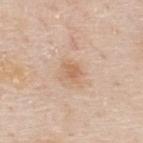Q: Was a biopsy performed?
A: catalogued during a skin exam; not biopsied
Q: What are the patient's age and sex?
A: male, aged 78–82
Q: What kind of image is this?
A: ~15 mm tile from a whole-body skin photo
Q: Where on the body is the lesion?
A: the upper back